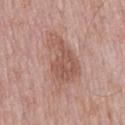Impression:
Imaged during a routine full-body skin examination; the lesion was not biopsied and no histopathology is available.
Clinical summary:
Automated image analysis of the tile measured an average lesion color of about L≈55 a*≈21 b*≈26 (CIELAB), roughly 9 lightness units darker than nearby skin, and a normalized lesion–skin contrast near 6.5. The analysis additionally found an automated nevus-likeness rating near 5 out of 100. The tile uses white-light illumination. A male subject about 75 years old. Approximately 7 mm at its widest. A lesion tile, about 15 mm wide, cut from a 3D total-body photograph. Located on the mid back.